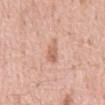follow-up: catalogued during a skin exam; not biopsied
anatomic site: the abdomen
patient: male, aged approximately 60
imaging modality: 15 mm crop, total-body photography